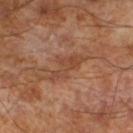biopsy status: no biopsy performed (imaged during a skin exam); patient: male, about 65 years old; acquisition: ~15 mm tile from a whole-body skin photo.